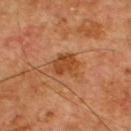Part of a total-body skin-imaging series; this lesion was reviewed on a skin check and was not flagged for biopsy. The tile uses cross-polarized illumination. A 15 mm crop from a total-body photograph taken for skin-cancer surveillance. Approximately 3 mm at its widest. The total-body-photography lesion software estimated an area of roughly 7 mm² and a symmetry-axis asymmetry near 0.25. It also reported border irregularity of about 2.5 on a 0–10 scale and a peripheral color-asymmetry measure near 1. A male subject in their mid-50s. On the chest.